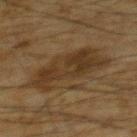Captured during whole-body skin photography for melanoma surveillance; the lesion was not biopsied. Cropped from a total-body skin-imaging series; the visible field is about 15 mm. The lesion is on the upper back. A male subject aged 58 to 62. Imaged with cross-polarized lighting. About 8 mm across. Automated tile analysis of the lesion measured a lesion color around L≈28 a*≈12 b*≈26 in CIELAB and roughly 7 lightness units darker than nearby skin. And it measured border irregularity of about 4.5 on a 0–10 scale, internal color variation of about 3.5 on a 0–10 scale, and peripheral color asymmetry of about 1.